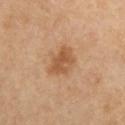Findings:
• workup: total-body-photography surveillance lesion; no biopsy
• location: the chest
• patient: female, aged 38–42
• diameter: ~4 mm (longest diameter)
• tile lighting: cross-polarized illumination
• image: 15 mm crop, total-body photography